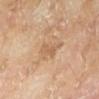| key | value |
|---|---|
| follow-up | total-body-photography surveillance lesion; no biopsy |
| image source | 15 mm crop, total-body photography |
| anatomic site | the left lower leg |
| diameter | ~3 mm (longest diameter) |
| patient | male, aged 63–67 |
| lighting | cross-polarized illumination |